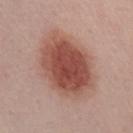Impression:
Part of a total-body skin-imaging series; this lesion was reviewed on a skin check and was not flagged for biopsy.
Clinical summary:
Automated image analysis of the tile measured a color-variation rating of about 5.5/10 and peripheral color asymmetry of about 1.5. A female subject aged approximately 55. A region of skin cropped from a whole-body photographic capture, roughly 15 mm wide. From the chest. About 8 mm across. Imaged with white-light lighting.